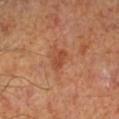{"biopsy_status": "not biopsied; imaged during a skin examination", "patient": {"sex": "male", "age_approx": 65}, "site": "left lower leg", "image": {"source": "total-body photography crop", "field_of_view_mm": 15}, "lighting": "cross-polarized", "lesion_size": {"long_diameter_mm_approx": 3.0}}